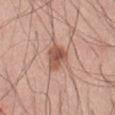| field | value |
|---|---|
| biopsy status | imaged on a skin check; not biopsied |
| subject | male, aged 53 to 57 |
| image | ~15 mm crop, total-body skin-cancer survey |
| lesion size | about 3.5 mm |
| site | the abdomen |
| tile lighting | white-light illumination |